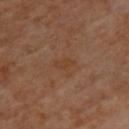Background:
A female patient aged 63–67. Captured under cross-polarized illumination. The lesion is located on the back. Measured at roughly 2.5 mm in maximum diameter. Automated tile analysis of the lesion measured a mean CIELAB color near L≈39 a*≈19 b*≈30, a lesion–skin lightness drop of about 4, and a normalized lesion–skin contrast near 5. And it measured border irregularity of about 3 on a 0–10 scale, internal color variation of about 1 on a 0–10 scale, and a peripheral color-asymmetry measure near 0.5. A 15 mm crop from a total-body photograph taken for skin-cancer surveillance.